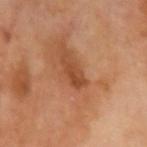{"biopsy_status": "not biopsied; imaged during a skin examination", "patient": {"sex": "male", "age_approx": 70}, "image": {"source": "total-body photography crop", "field_of_view_mm": 15}, "site": "left upper arm"}